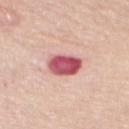A roughly 15 mm field-of-view crop from a total-body skin photograph. The lesion is on the mid back. A female patient in their 70s.